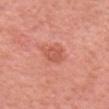Recorded during total-body skin imaging; not selected for excision or biopsy. Longest diameter approximately 2.5 mm. A 15 mm close-up tile from a total-body photography series done for melanoma screening. The lesion is on the head or neck. A female patient aged 48–52.